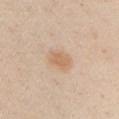follow-up: imaged on a skin check; not biopsied | body site: the left upper arm | acquisition: ~15 mm crop, total-body skin-cancer survey | patient: male, roughly 60 years of age | illumination: white-light | lesion diameter: ~3 mm (longest diameter).